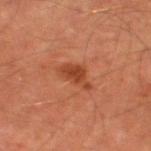Assessment: Imaged during a routine full-body skin examination; the lesion was not biopsied and no histopathology is available. Clinical summary: This is a cross-polarized tile. Located on the left thigh. A close-up tile cropped from a whole-body skin photograph, about 15 mm across. A male patient, aged 63–67.